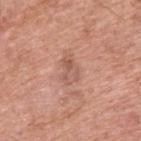Findings:
• workup: no biopsy performed (imaged during a skin exam)
• location: the upper back
• image: total-body-photography crop, ~15 mm field of view
• subject: male, aged 53 to 57
• tile lighting: white-light
• lesion diameter: about 3 mm